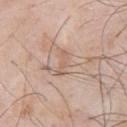This lesion was catalogued during total-body skin photography and was not selected for biopsy. A male subject roughly 55 years of age. On the chest. The lesion-visualizer software estimated a footprint of about 3 mm², a shape eccentricity near 0.95, and a shape-asymmetry score of about 0.45 (0 = symmetric). And it measured a lesion color around L≈61 a*≈18 b*≈28 in CIELAB. The software also gave border irregularity of about 5.5 on a 0–10 scale, a color-variation rating of about 0/10, and peripheral color asymmetry of about 0. About 3 mm across. This is a white-light tile. A 15 mm close-up tile from a total-body photography series done for melanoma screening.